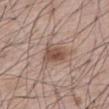The lesion was tiled from a total-body skin photograph and was not biopsied.